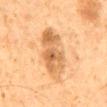biopsy status — imaged on a skin check; not biopsied | subject — male, aged around 60 | site — the mid back | imaging modality — ~15 mm crop, total-body skin-cancer survey | size — about 9 mm | automated lesion analysis — a footprint of about 19 mm², a shape eccentricity near 0.95, and a shape-asymmetry score of about 0.15 (0 = symmetric); a within-lesion color-variation index near 6.5/10 and a peripheral color-asymmetry measure near 2.5.